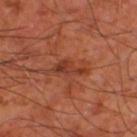Findings:
* follow-up — total-body-photography surveillance lesion; no biopsy
* image source — 15 mm crop, total-body photography
* site — the right thigh
* tile lighting — cross-polarized illumination
* subject — about 65 years old